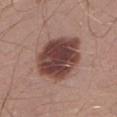| key | value |
|---|---|
| tile lighting | white-light |
| diameter | ~6.5 mm (longest diameter) |
| body site | the left lower leg |
| acquisition | total-body-photography crop, ~15 mm field of view |
| automated lesion analysis | a footprint of about 26 mm², an outline eccentricity of about 0.6 (0 = round, 1 = elongated), and a shape-asymmetry score of about 0.15 (0 = symmetric); an average lesion color of about L≈42 a*≈21 b*≈22 (CIELAB), a lesion–skin lightness drop of about 17, and a normalized lesion–skin contrast near 12.5; border irregularity of about 1.5 on a 0–10 scale, internal color variation of about 5 on a 0–10 scale, and radial color variation of about 1.5 |
| subject | male, aged 28 to 32 |